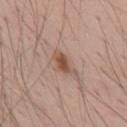Captured during whole-body skin photography for melanoma surveillance; the lesion was not biopsied.
Cropped from a total-body skin-imaging series; the visible field is about 15 mm.
The lesion is on the upper back.
A male patient, aged approximately 70.
Captured under white-light illumination.
The lesion-visualizer software estimated a lesion color around L≈52 a*≈19 b*≈27 in CIELAB, about 11 CIELAB-L* units darker than the surrounding skin, and a normalized border contrast of about 8. And it measured a nevus-likeness score of about 95/100 and lesion-presence confidence of about 100/100.
Longest diameter approximately 4 mm.